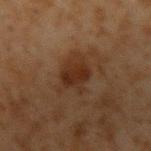The lesion was photographed on a routine skin check and not biopsied; there is no pathology result. The lesion is located on the left upper arm. The lesion's longest dimension is about 4 mm. A lesion tile, about 15 mm wide, cut from a 3D total-body photograph. A male patient roughly 45 years of age.